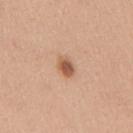  lesion_size:
    long_diameter_mm_approx: 2.5
  patient:
    sex: female
    age_approx: 40
  site: right upper arm
  lighting: white-light
  image:
    source: total-body photography crop
    field_of_view_mm: 15